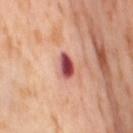Imaged during a routine full-body skin examination; the lesion was not biopsied and no histopathology is available. Imaged with cross-polarized lighting. This image is a 15 mm lesion crop taken from a total-body photograph. The lesion is located on the leg. The patient is a female in their mid-50s.